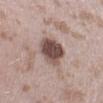<tbp_lesion>
  <biopsy_status>not biopsied; imaged during a skin examination</biopsy_status>
  <lesion_size>
    <long_diameter_mm_approx>4.5</long_diameter_mm_approx>
  </lesion_size>
  <image>
    <source>total-body photography crop</source>
    <field_of_view_mm>15</field_of_view_mm>
  </image>
  <patient>
    <sex>female</sex>
    <age_approx>25</age_approx>
  </patient>
  <automated_metrics>
    <cielab_L>49</cielab_L>
    <cielab_a>17</cielab_a>
    <cielab_b>21</cielab_b>
    <vs_skin_darker_L>17.0</vs_skin_darker_L>
    <vs_skin_contrast_norm>11.5</vs_skin_contrast_norm>
  </automated_metrics>
  <site>leg</site>
  <lighting>white-light</lighting>
</tbp_lesion>